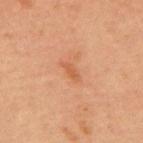Captured during whole-body skin photography for melanoma surveillance; the lesion was not biopsied.
Automated tile analysis of the lesion measured a lesion area of about 2.5 mm², an eccentricity of roughly 0.9, and two-axis asymmetry of about 0.3. And it measured a mean CIELAB color near L≈52 a*≈24 b*≈36, about 7 CIELAB-L* units darker than the surrounding skin, and a normalized lesion–skin contrast near 5.5. The analysis additionally found a peripheral color-asymmetry measure near 0. And it measured a lesion-detection confidence of about 100/100.
This is a cross-polarized tile.
Cropped from a total-body skin-imaging series; the visible field is about 15 mm.
The subject is a male approximately 60 years of age.
The lesion is located on the chest.